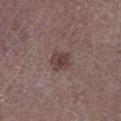Imaged during a routine full-body skin examination; the lesion was not biopsied and no histopathology is available.
This image is a 15 mm lesion crop taken from a total-body photograph.
The total-body-photography lesion software estimated an area of roughly 5.5 mm² and a shape-asymmetry score of about 0.25 (0 = symmetric). The analysis additionally found an automated nevus-likeness rating near 40 out of 100.
Located on the right lower leg.
Approximately 2.5 mm at its widest.
A male subject aged around 70.
Captured under white-light illumination.